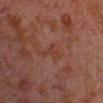This lesion was catalogued during total-body skin photography and was not selected for biopsy. The tile uses cross-polarized illumination. Cropped from a total-body skin-imaging series; the visible field is about 15 mm. About 2.5 mm across. Automated tile analysis of the lesion measured a lesion color around L≈31 a*≈20 b*≈24 in CIELAB, roughly 4 lightness units darker than nearby skin, and a normalized border contrast of about 5. It also reported border irregularity of about 7.5 on a 0–10 scale, a within-lesion color-variation index near 0/10, and radial color variation of about 0. The software also gave an automated nevus-likeness rating near 0 out of 100 and a detector confidence of about 95 out of 100 that the crop contains a lesion. The patient is a male roughly 30 years of age. The lesion is on the right forearm.